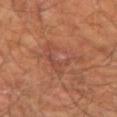Q: Was a biopsy performed?
A: no biopsy performed (imaged during a skin exam)
Q: What lighting was used for the tile?
A: cross-polarized
Q: Who is the patient?
A: male, in their mid- to late 60s
Q: What is the imaging modality?
A: 15 mm crop, total-body photography
Q: How large is the lesion?
A: ~5.5 mm (longest diameter)
Q: Automated lesion metrics?
A: roughly 6 lightness units darker than nearby skin and a normalized border contrast of about 5
Q: Where on the body is the lesion?
A: the arm